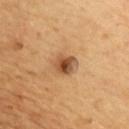<record>
<biopsy_status>not biopsied; imaged during a skin examination</biopsy_status>
<site>chest</site>
<image>
  <source>total-body photography crop</source>
  <field_of_view_mm>15</field_of_view_mm>
</image>
<patient>
  <sex>male</sex>
  <age_approx>45</age_approx>
</patient>
<lighting>cross-polarized</lighting>
</record>